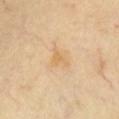biopsy status: catalogued during a skin exam; not biopsied
image-analysis metrics: an automated nevus-likeness rating near 0 out of 100 and a detector confidence of about 100 out of 100 that the crop contains a lesion
acquisition: total-body-photography crop, ~15 mm field of view
tile lighting: cross-polarized
site: the front of the torso
patient: female, aged approximately 60